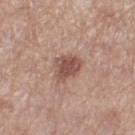Assessment: Imaged during a routine full-body skin examination; the lesion was not biopsied and no histopathology is available. Background: A female patient roughly 55 years of age. Longest diameter approximately 3.5 mm. Imaged with white-light lighting. A 15 mm close-up tile from a total-body photography series done for melanoma screening. Located on the leg.